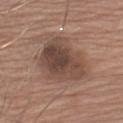Imaged during a routine full-body skin examination; the lesion was not biopsied and no histopathology is available.
A roughly 15 mm field-of-view crop from a total-body skin photograph.
A male patient, aged 63 to 67.
The lesion is on the left upper arm.